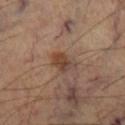Clinical summary:
A male subject aged 53–57. This is a cross-polarized tile. The lesion is located on the right lower leg. About 2.5 mm across. A close-up tile cropped from a whole-body skin photograph, about 15 mm across. Automated image analysis of the tile measured a lesion area of about 4.5 mm², an eccentricity of roughly 0.65, and two-axis asymmetry of about 0.25. It also reported an average lesion color of about L≈42 a*≈18 b*≈28 (CIELAB), a lesion–skin lightness drop of about 9, and a normalized border contrast of about 8. The software also gave a border-irregularity rating of about 2.5/10, a within-lesion color-variation index near 4.5/10, and a peripheral color-asymmetry measure near 1.5. And it measured a classifier nevus-likeness of about 85/100 and a lesion-detection confidence of about 100/100.